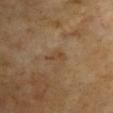Impression: Imaged during a routine full-body skin examination; the lesion was not biopsied and no histopathology is available. Acquisition and patient details: Cropped from a total-body skin-imaging series; the visible field is about 15 mm. The lesion is on the front of the torso. Longest diameter approximately 2.5 mm. Imaged with cross-polarized lighting. A female patient, in their 60s.